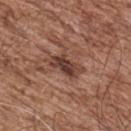This lesion was catalogued during total-body skin photography and was not selected for biopsy. Located on the upper back. Captured under white-light illumination. The patient is a male aged around 55. A lesion tile, about 15 mm wide, cut from a 3D total-body photograph. Longest diameter approximately 4 mm.